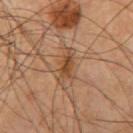Clinical impression:
This lesion was catalogued during total-body skin photography and was not selected for biopsy.
Acquisition and patient details:
Automated tile analysis of the lesion measured a lesion color around L≈46 a*≈19 b*≈33 in CIELAB, a lesion–skin lightness drop of about 9, and a normalized border contrast of about 7.5. The analysis additionally found a detector confidence of about 95 out of 100 that the crop contains a lesion. The lesion is located on the right upper arm. Cropped from a whole-body photographic skin survey; the tile spans about 15 mm. A male patient, aged around 60.